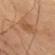  biopsy_status: not biopsied; imaged during a skin examination